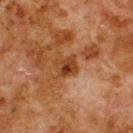Part of a total-body skin-imaging series; this lesion was reviewed on a skin check and was not flagged for biopsy.
An algorithmic analysis of the crop reported an area of roughly 5 mm², an outline eccentricity of about 0.6 (0 = round, 1 = elongated), and a symmetry-axis asymmetry near 0.25. It also reported a lesion color around L≈31 a*≈21 b*≈30 in CIELAB, a lesion–skin lightness drop of about 9, and a normalized border contrast of about 8.5. And it measured a classifier nevus-likeness of about 5/100 and a detector confidence of about 100 out of 100 that the crop contains a lesion.
The subject is a male aged 78–82.
A region of skin cropped from a whole-body photographic capture, roughly 15 mm wide.
Located on the upper back.